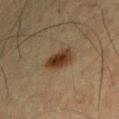patient = male, aged 58–62 | tile lighting = cross-polarized | diameter = about 3.5 mm | location = the right forearm | acquisition = ~15 mm tile from a whole-body skin photo.